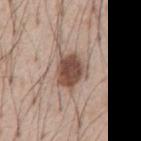Case summary:
– follow-up: catalogued during a skin exam; not biopsied
– image: 15 mm crop, total-body photography
– site: the abdomen
– subject: male, approximately 55 years of age